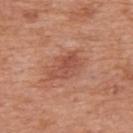Part of a total-body skin-imaging series; this lesion was reviewed on a skin check and was not flagged for biopsy.
The recorded lesion diameter is about 4 mm.
A 15 mm crop from a total-body photograph taken for skin-cancer surveillance.
The subject is a male aged 68–72.
From the back.
This is a white-light tile.
The total-body-photography lesion software estimated an area of roughly 8 mm², an outline eccentricity of about 0.85 (0 = round, 1 = elongated), and a shape-asymmetry score of about 0.3 (0 = symmetric). It also reported a mean CIELAB color near L≈51 a*≈26 b*≈31 and roughly 8 lightness units darker than nearby skin.